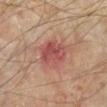No biopsy was performed on this lesion — it was imaged during a full skin examination and was not determined to be concerning.
Cropped from a whole-body photographic skin survey; the tile spans about 15 mm.
On the left lower leg.
The subject is in their mid-50s.
Captured under cross-polarized illumination.
Automated image analysis of the tile measured a footprint of about 11 mm², an outline eccentricity of about 0.6 (0 = round, 1 = elongated), and a shape-asymmetry score of about 0.2 (0 = symmetric). The analysis additionally found a border-irregularity rating of about 2/10, a within-lesion color-variation index near 5/10, and peripheral color asymmetry of about 2. And it measured an automated nevus-likeness rating near 0 out of 100 and a lesion-detection confidence of about 100/100.
About 4.5 mm across.